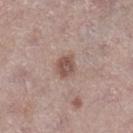Part of a total-body skin-imaging series; this lesion was reviewed on a skin check and was not flagged for biopsy.
Captured under white-light illumination.
Measured at roughly 3 mm in maximum diameter.
From the right lower leg.
Cropped from a total-body skin-imaging series; the visible field is about 15 mm.
A female patient, aged approximately 65.